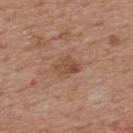No biopsy was performed on this lesion — it was imaged during a full skin examination and was not determined to be concerning.
The tile uses white-light illumination.
Longest diameter approximately 3 mm.
Located on the upper back.
A male patient, about 55 years old.
Cropped from a total-body skin-imaging series; the visible field is about 15 mm.